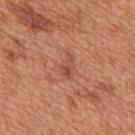Q: Was this lesion biopsied?
A: imaged on a skin check; not biopsied
Q: What kind of image is this?
A: ~15 mm crop, total-body skin-cancer survey
Q: What is the anatomic site?
A: the back
Q: How was the tile lit?
A: white-light
Q: Patient demographics?
A: male, aged 63–67